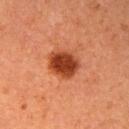| feature | finding |
|---|---|
| anatomic site | the right upper arm |
| patient | female, aged 58 to 62 |
| acquisition | ~15 mm tile from a whole-body skin photo |